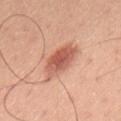Q: Is there a histopathology result?
A: imaged on a skin check; not biopsied
Q: Illumination type?
A: white-light illumination
Q: Lesion location?
A: the upper back
Q: What kind of image is this?
A: total-body-photography crop, ~15 mm field of view
Q: Who is the patient?
A: male, aged around 45